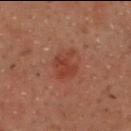The lesion was tiled from a total-body skin photograph and was not biopsied. A male subject aged 48–52. The total-body-photography lesion software estimated a footprint of about 6.5 mm², an eccentricity of roughly 0.6, and a symmetry-axis asymmetry near 0.3. The software also gave a border-irregularity index near 3.5/10 and a color-variation rating of about 2/10. A roughly 15 mm field-of-view crop from a total-body skin photograph. The lesion is located on the chest.